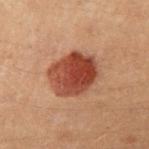{"biopsy_status": "not biopsied; imaged during a skin examination", "lighting": "cross-polarized", "patient": {"sex": "female", "age_approx": 30}, "lesion_size": {"long_diameter_mm_approx": 5.5}, "image": {"source": "total-body photography crop", "field_of_view_mm": 15}, "site": "left arm"}